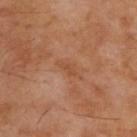biopsy status: no biopsy performed (imaged during a skin exam) | subject: male, aged 53–57 | lesion diameter: about 2.5 mm | imaging modality: 15 mm crop, total-body photography | illumination: cross-polarized | site: the back.